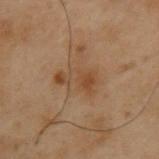Captured during whole-body skin photography for melanoma surveillance; the lesion was not biopsied. Located on the upper back. Cropped from a whole-body photographic skin survey; the tile spans about 15 mm. A male patient aged around 55.